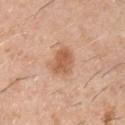TBP lesion metrics: a footprint of about 7.5 mm², an eccentricity of roughly 0.65, and a shape-asymmetry score of about 0.25 (0 = symmetric); a color-variation rating of about 3.5/10 and peripheral color asymmetry of about 1
illumination: white-light
anatomic site: the chest
subject: male, approximately 60 years of age
image source: ~15 mm crop, total-body skin-cancer survey
lesion diameter: about 3.5 mm